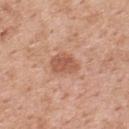{
  "biopsy_status": "not biopsied; imaged during a skin examination",
  "site": "upper back",
  "lighting": "white-light",
  "image": {
    "source": "total-body photography crop",
    "field_of_view_mm": 15
  },
  "patient": {
    "sex": "female",
    "age_approx": 50
  }
}